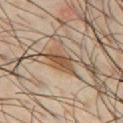Case summary:
• workup — total-body-photography surveillance lesion; no biopsy
• image source — 15 mm crop, total-body photography
• patient — male, aged 38 to 42
• automated metrics — a lesion area of about 7 mm², an eccentricity of roughly 0.85, and a shape-asymmetry score of about 0.3 (0 = symmetric); a border-irregularity index near 3.5/10, internal color variation of about 3.5 on a 0–10 scale, and peripheral color asymmetry of about 1.5
• tile lighting — cross-polarized illumination
• site — the chest
• lesion size — about 4 mm